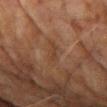Impression:
No biopsy was performed on this lesion — it was imaged during a full skin examination and was not determined to be concerning.
Image and clinical context:
Measured at roughly 2.5 mm in maximum diameter. A 15 mm close-up extracted from a 3D total-body photography capture. The patient is a male aged 73–77. Located on the chest. Captured under cross-polarized illumination.